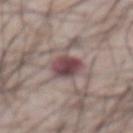Clinical impression: The lesion was photographed on a routine skin check and not biopsied; there is no pathology result. Image and clinical context: An algorithmic analysis of the crop reported a lesion area of about 7.5 mm², an outline eccentricity of about 0.6 (0 = round, 1 = elongated), and two-axis asymmetry of about 0.25. It also reported a mean CIELAB color near L≈44 a*≈20 b*≈15 and a normalized border contrast of about 11.5. And it measured a classifier nevus-likeness of about 60/100 and a detector confidence of about 100 out of 100 that the crop contains a lesion. Imaged with white-light lighting. About 3.5 mm across. A male patient roughly 65 years of age. A 15 mm close-up extracted from a 3D total-body photography capture. On the abdomen.